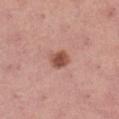Findings:
- biopsy status · imaged on a skin check; not biopsied
- body site · the leg
- image · ~15 mm crop, total-body skin-cancer survey
- illumination · white-light illumination
- patient · female, aged around 40
- diameter · ≈2.5 mm